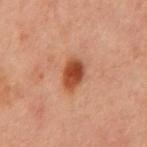biopsy_status: not biopsied; imaged during a skin examination
patient:
  sex: male
  age_approx: 60
image:
  source: total-body photography crop
  field_of_view_mm: 15
lesion_size:
  long_diameter_mm_approx: 3.5
site: front of the torso